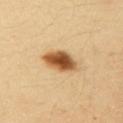Imaged during a routine full-body skin examination; the lesion was not biopsied and no histopathology is available. A female subject, aged 33 to 37. A 15 mm crop from a total-body photograph taken for skin-cancer surveillance. From the arm. Measured at roughly 4.5 mm in maximum diameter.